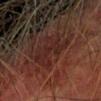notes=imaged on a skin check; not biopsied
body site=the left forearm
image source=total-body-photography crop, ~15 mm field of view
lesion diameter=≈4.5 mm
lighting=cross-polarized
subject=male, aged around 75